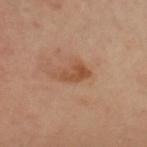{
  "image": {
    "source": "total-body photography crop",
    "field_of_view_mm": 15
  },
  "automated_metrics": {
    "border_irregularity_0_10": 4.5,
    "color_variation_0_10": 2.5,
    "nevus_likeness_0_100": 20,
    "lesion_detection_confidence_0_100": 100
  },
  "lighting": "cross-polarized",
  "patient": {
    "sex": "female",
    "age_approx": 50
  },
  "site": "back"
}